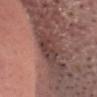Captured during whole-body skin photography for melanoma surveillance; the lesion was not biopsied.
Imaged with white-light lighting.
About 3.5 mm across.
A male patient aged approximately 45.
Located on the head or neck.
Cropped from a whole-body photographic skin survey; the tile spans about 15 mm.